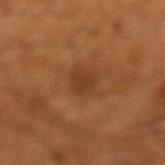The lesion was tiled from a total-body skin photograph and was not biopsied. On the left lower leg. Automated tile analysis of the lesion measured a lesion area of about 4.5 mm², an outline eccentricity of about 0.75 (0 = round, 1 = elongated), and a symmetry-axis asymmetry near 0.25. The analysis additionally found an average lesion color of about L≈37 a*≈21 b*≈33 (CIELAB), roughly 7 lightness units darker than nearby skin, and a normalized lesion–skin contrast near 6.5. The analysis additionally found a border-irregularity index near 2/10, a color-variation rating of about 1.5/10, and peripheral color asymmetry of about 0.5. About 2.5 mm across. A 15 mm close-up tile from a total-body photography series done for melanoma screening. The patient is a male aged 63 to 67. Imaged with cross-polarized lighting.